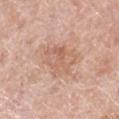Imaged during a routine full-body skin examination; the lesion was not biopsied and no histopathology is available.
Imaged with white-light lighting.
Cropped from a whole-body photographic skin survey; the tile spans about 15 mm.
A female subject, aged approximately 65.
About 4.5 mm across.
Located on the right lower leg.